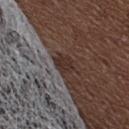Impression:
This lesion was catalogued during total-body skin photography and was not selected for biopsy.
Background:
A female patient aged approximately 65. On the head or neck. A roughly 15 mm field-of-view crop from a total-body skin photograph. Imaged with white-light lighting. The total-body-photography lesion software estimated a lesion area of about 4.5 mm². And it measured a border-irregularity rating of about 2.5/10 and peripheral color asymmetry of about 0.5. It also reported a nevus-likeness score of about 0/100 and a lesion-detection confidence of about 55/100.